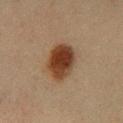The lesion was tiled from a total-body skin photograph and was not biopsied. A roughly 15 mm field-of-view crop from a total-body skin photograph. A male patient, about 65 years old. Longest diameter approximately 5 mm. The total-body-photography lesion software estimated an average lesion color of about L≈31 a*≈16 b*≈26 (CIELAB), roughly 12 lightness units darker than nearby skin, and a normalized border contrast of about 12. The software also gave radial color variation of about 1. Imaged with cross-polarized lighting. The lesion is on the leg.